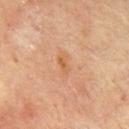Captured under cross-polarized illumination.
A male patient, in their mid-60s.
The recorded lesion diameter is about 2.5 mm.
The lesion is on the chest.
This image is a 15 mm lesion crop taken from a total-body photograph.
The total-body-photography lesion software estimated a detector confidence of about 100 out of 100 that the crop contains a lesion.